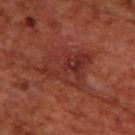Clinical impression:
Part of a total-body skin-imaging series; this lesion was reviewed on a skin check and was not flagged for biopsy.